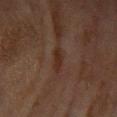biopsy status: no biopsy performed (imaged during a skin exam) | image source: ~15 mm tile from a whole-body skin photo | patient: female, in their 80s | site: the arm | tile lighting: cross-polarized.